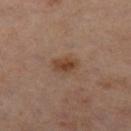{"biopsy_status": "not biopsied; imaged during a skin examination", "image": {"source": "total-body photography crop", "field_of_view_mm": 15}, "lesion_size": {"long_diameter_mm_approx": 2.5}, "patient": {"sex": "male", "age_approx": 60}, "site": "right thigh", "automated_metrics": {"nevus_likeness_0_100": 80, "lesion_detection_confidence_0_100": 100}}